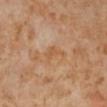  biopsy_status: not biopsied; imaged during a skin examination
  patient:
    sex: female
    age_approx: 50
  lighting: cross-polarized
  site: right lower leg
  image:
    source: total-body photography crop
    field_of_view_mm: 15
  automated_metrics:
    area_mm2_approx: 4.0
    eccentricity: 0.75
    shape_asymmetry: 0.5
    color_variation_0_10: 1.0
    peripheral_color_asymmetry: 0.5
  lesion_size:
    long_diameter_mm_approx: 3.0